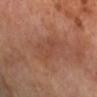Context: Cropped from a total-body skin-imaging series; the visible field is about 15 mm. The lesion is located on the left forearm. A male patient aged 68 to 72.